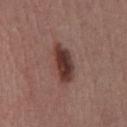| feature | finding |
|---|---|
| workup | no biopsy performed (imaged during a skin exam) |